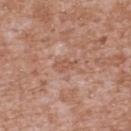Clinical impression: Imaged during a routine full-body skin examination; the lesion was not biopsied and no histopathology is available. Acquisition and patient details: A male subject, roughly 45 years of age. A close-up tile cropped from a whole-body skin photograph, about 15 mm across. From the upper back.